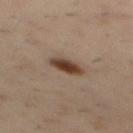notes: catalogued during a skin exam; not biopsied
size: ~3.5 mm (longest diameter)
image-analysis metrics: a lesion color around L≈40 a*≈16 b*≈26 in CIELAB and a lesion–skin lightness drop of about 13; a nevus-likeness score of about 100/100 and lesion-presence confidence of about 100/100
location: the back
image source: 15 mm crop, total-body photography
subject: male, aged 38 to 42
illumination: cross-polarized